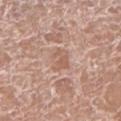Assessment:
Recorded during total-body skin imaging; not selected for excision or biopsy.
Clinical summary:
A female patient, in their mid-70s. This image is a 15 mm lesion crop taken from a total-body photograph. From the right lower leg. The lesion's longest dimension is about 3 mm.